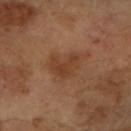{
  "biopsy_status": "not biopsied; imaged during a skin examination",
  "image": {
    "source": "total-body photography crop",
    "field_of_view_mm": 15
  },
  "automated_metrics": {
    "area_mm2_approx": 9.0,
    "eccentricity": 0.7,
    "shape_asymmetry": 0.35,
    "cielab_L": 41,
    "cielab_a": 21,
    "cielab_b": 33,
    "vs_skin_darker_L": 7.0,
    "vs_skin_contrast_norm": 6.0,
    "border_irregularity_0_10": 4.0,
    "color_variation_0_10": 2.0
  },
  "patient": {
    "sex": "female",
    "age_approx": 70
  },
  "site": "arm"
}